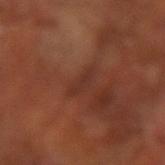Cropped from a whole-body photographic skin survey; the tile spans about 15 mm. The lesion is located on the left arm. A male subject aged 63–67. The lesion's longest dimension is about 3 mm. This is a cross-polarized tile.